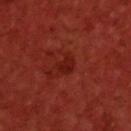{
  "biopsy_status": "not biopsied; imaged during a skin examination",
  "image": {
    "source": "total-body photography crop",
    "field_of_view_mm": 15
  },
  "automated_metrics": {
    "area_mm2_approx": 4.0,
    "eccentricity": 0.7,
    "shape_asymmetry": 0.35,
    "cielab_L": 22,
    "cielab_a": 30,
    "cielab_b": 28,
    "vs_skin_darker_L": 6.0,
    "vs_skin_contrast_norm": 6.0
  },
  "lesion_size": {
    "long_diameter_mm_approx": 2.5
  },
  "patient": {
    "sex": "male",
    "age_approx": 60
  },
  "site": "upper back",
  "lighting": "cross-polarized"
}